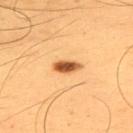Recorded during total-body skin imaging; not selected for excision or biopsy.
Automated image analysis of the tile measured a lesion color around L≈56 a*≈26 b*≈44 in CIELAB, about 20 CIELAB-L* units darker than the surrounding skin, and a normalized border contrast of about 12. And it measured a border-irregularity rating of about 2/10, internal color variation of about 3.5 on a 0–10 scale, and radial color variation of about 1.
The tile uses cross-polarized illumination.
A lesion tile, about 15 mm wide, cut from a 3D total-body photograph.
Measured at roughly 3.5 mm in maximum diameter.
A male patient approximately 55 years of age.
From the upper back.